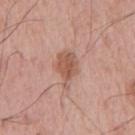No biopsy was performed on this lesion — it was imaged during a full skin examination and was not determined to be concerning.
The patient is a male roughly 65 years of age.
Captured under white-light illumination.
An algorithmic analysis of the crop reported a footprint of about 7.5 mm², a shape eccentricity near 0.8, and a shape-asymmetry score of about 0.35 (0 = symmetric). The analysis additionally found a classifier nevus-likeness of about 60/100.
A 15 mm close-up extracted from a 3D total-body photography capture.
About 4.5 mm across.
The lesion is on the mid back.